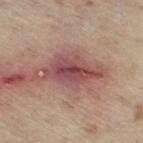workup: imaged on a skin check; not biopsied | subject: male, aged approximately 70 | image source: 15 mm crop, total-body photography | anatomic site: the right thigh.